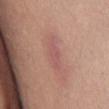Q: Was a biopsy performed?
A: no biopsy performed (imaged during a skin exam)
Q: How was the tile lit?
A: white-light illumination
Q: What did automated image analysis measure?
A: a footprint of about 7 mm², an outline eccentricity of about 0.9 (0 = round, 1 = elongated), and a shape-asymmetry score of about 0.25 (0 = symmetric); a mean CIELAB color near L≈55 a*≈24 b*≈23, a lesion–skin lightness drop of about 6, and a normalized lesion–skin contrast near 5; internal color variation of about 1.5 on a 0–10 scale and peripheral color asymmetry of about 0.5
Q: What is the lesion's diameter?
A: ≈4.5 mm
Q: What is the imaging modality?
A: ~15 mm crop, total-body skin-cancer survey
Q: Who is the patient?
A: male, in their mid- to late 50s